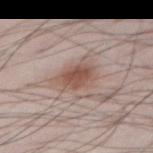No biopsy was performed on this lesion — it was imaged during a full skin examination and was not determined to be concerning.
A male subject, aged around 40.
On the right thigh.
Cropped from a total-body skin-imaging series; the visible field is about 15 mm.